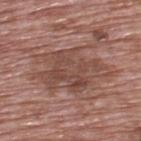Assessment: Part of a total-body skin-imaging series; this lesion was reviewed on a skin check and was not flagged for biopsy. Acquisition and patient details: A 15 mm close-up tile from a total-body photography series done for melanoma screening. A male patient, aged 68 to 72. From the upper back.